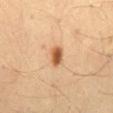Captured during whole-body skin photography for melanoma surveillance; the lesion was not biopsied.
The lesion-visualizer software estimated a lesion color around L≈53 a*≈22 b*≈36 in CIELAB, about 14 CIELAB-L* units darker than the surrounding skin, and a lesion-to-skin contrast of about 10 (normalized; higher = more distinct).
About 2.5 mm across.
This image is a 15 mm lesion crop taken from a total-body photograph.
This is a cross-polarized tile.
On the mid back.
A male patient approximately 35 years of age.